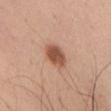biopsy status: catalogued during a skin exam; not biopsied | imaging modality: total-body-photography crop, ~15 mm field of view | lighting: white-light | location: the chest | size: ~4 mm (longest diameter) | TBP lesion metrics: an area of roughly 7.5 mm², an eccentricity of roughly 0.75, and two-axis asymmetry of about 0.15; lesion-presence confidence of about 100/100 | patient: male, roughly 35 years of age.